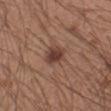<tbp_lesion>
<automated_metrics>
  <shape_asymmetry>0.2</shape_asymmetry>
  <border_irregularity_0_10>2.0</border_irregularity_0_10>
  <color_variation_0_10>3.0</color_variation_0_10>
  <peripheral_color_asymmetry>1.0</peripheral_color_asymmetry>
  <lesion_detection_confidence_0_100>100</lesion_detection_confidence_0_100>
</automated_metrics>
<lesion_size>
  <long_diameter_mm_approx>2.5</long_diameter_mm_approx>
</lesion_size>
<image>
  <source>total-body photography crop</source>
  <field_of_view_mm>15</field_of_view_mm>
</image>
<lighting>white-light</lighting>
<site>arm</site>
<patient>
  <sex>male</sex>
  <age_approx>20</age_approx>
</patient>
</tbp_lesion>